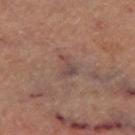This lesion was catalogued during total-body skin photography and was not selected for biopsy.
The lesion is on the left thigh.
This image is a 15 mm lesion crop taken from a total-body photograph.
Imaged with cross-polarized lighting.
The patient is a male aged approximately 60.
Automated tile analysis of the lesion measured a lesion area of about 4 mm², an outline eccentricity of about 0.65 (0 = round, 1 = elongated), and a symmetry-axis asymmetry near 0.5. It also reported about 6 CIELAB-L* units darker than the surrounding skin and a normalized border contrast of about 6. The analysis additionally found internal color variation of about 2.5 on a 0–10 scale and radial color variation of about 1.